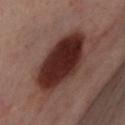image = ~15 mm crop, total-body skin-cancer survey
size = about 8.5 mm
subject = female, in their mid- to late 50s
illumination = cross-polarized
anatomic site = the leg